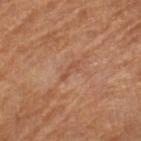Case summary:
* workup · total-body-photography surveillance lesion; no biopsy
* imaging modality · ~15 mm tile from a whole-body skin photo
* lesion diameter · ~2.5 mm (longest diameter)
* TBP lesion metrics · a lesion area of about 1.5 mm², an eccentricity of roughly 0.95, and a shape-asymmetry score of about 0.4 (0 = symmetric); a border-irregularity index near 5.5/10, a color-variation rating of about 0/10, and peripheral color asymmetry of about 0; a nevus-likeness score of about 0/100 and lesion-presence confidence of about 95/100
* body site · the right lower leg
* subject · male, aged approximately 85 or older